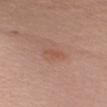Q: Was a biopsy performed?
A: total-body-photography surveillance lesion; no biopsy
Q: Automated lesion metrics?
A: a lesion color around L≈53 a*≈23 b*≈29 in CIELAB, about 6 CIELAB-L* units darker than the surrounding skin, and a normalized border contrast of about 5; a nevus-likeness score of about 15/100 and a detector confidence of about 100 out of 100 that the crop contains a lesion
Q: How was the tile lit?
A: white-light
Q: What is the imaging modality?
A: ~15 mm crop, total-body skin-cancer survey
Q: What is the anatomic site?
A: the left upper arm
Q: What are the patient's age and sex?
A: female, about 45 years old